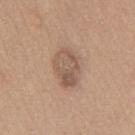workup: no biopsy performed (imaged during a skin exam); illumination: white-light; anatomic site: the mid back; automated metrics: a lesion color around L≈55 a*≈16 b*≈27 in CIELAB, roughly 9 lightness units darker than nearby skin, and a normalized lesion–skin contrast near 6.5; patient: male, roughly 60 years of age; diameter: ~4.5 mm (longest diameter); imaging modality: ~15 mm crop, total-body skin-cancer survey.